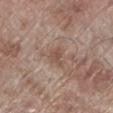Assessment:
No biopsy was performed on this lesion — it was imaged during a full skin examination and was not determined to be concerning.
Image and clinical context:
On the left lower leg. This is a white-light tile. A 15 mm close-up tile from a total-body photography series done for melanoma screening. A male patient in their 70s.